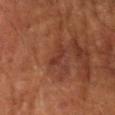Captured during whole-body skin photography for melanoma surveillance; the lesion was not biopsied.
The patient is a female approximately 80 years of age.
The recorded lesion diameter is about 3 mm.
From the right forearm.
A 15 mm close-up tile from a total-body photography series done for melanoma screening.
Imaged with cross-polarized lighting.